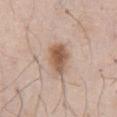No biopsy was performed on this lesion — it was imaged during a full skin examination and was not determined to be concerning. Measured at roughly 4 mm in maximum diameter. The lesion is located on the front of the torso. Imaged with white-light lighting. A male patient roughly 55 years of age. A 15 mm crop from a total-body photograph taken for skin-cancer surveillance.